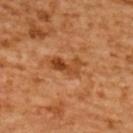Part of a total-body skin-imaging series; this lesion was reviewed on a skin check and was not flagged for biopsy. Located on the upper back. A female patient, in their mid-50s. A close-up tile cropped from a whole-body skin photograph, about 15 mm across. An algorithmic analysis of the crop reported a lesion area of about 6 mm², a shape eccentricity near 0.9, and a symmetry-axis asymmetry near 0.35. It also reported border irregularity of about 4.5 on a 0–10 scale and a color-variation rating of about 7.5/10. This is a cross-polarized tile. The recorded lesion diameter is about 4 mm.